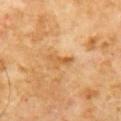follow-up = no biopsy performed (imaged during a skin exam); subject = male, in their 60s; anatomic site = the chest; size = ~3 mm (longest diameter); image = ~15 mm tile from a whole-body skin photo; lighting = cross-polarized.